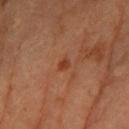notes = catalogued during a skin exam; not biopsied
patient = female, roughly 65 years of age
automated metrics = a border-irregularity rating of about 2/10 and internal color variation of about 2.5 on a 0–10 scale
site = the arm
lesion diameter = ~1.5 mm (longest diameter)
image = ~15 mm crop, total-body skin-cancer survey